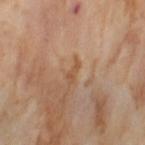  biopsy_status: not biopsied; imaged during a skin examination
  automated_metrics:
    eccentricity: 0.95
    shape_asymmetry: 0.45
    cielab_L: 53
    cielab_a: 20
    cielab_b: 34
    vs_skin_darker_L: 7.0
    vs_skin_contrast_norm: 6.0
    border_irregularity_0_10: 5.0
    color_variation_0_10: 0.0
    peripheral_color_asymmetry: 0.0
  image:
    source: total-body photography crop
    field_of_view_mm: 15
  site: left thigh
  lighting: cross-polarized
  patient:
    sex: female
    age_approx: 55